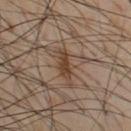workup: no biopsy performed (imaged during a skin exam)
imaging modality: 15 mm crop, total-body photography
illumination: cross-polarized
TBP lesion metrics: a lesion color around L≈42 a*≈16 b*≈28 in CIELAB, roughly 9 lightness units darker than nearby skin, and a lesion-to-skin contrast of about 8.5 (normalized; higher = more distinct); a nevus-likeness score of about 10/100 and a detector confidence of about 100 out of 100 that the crop contains a lesion
body site: the chest
size: ~4 mm (longest diameter)
patient: male, aged 53–57